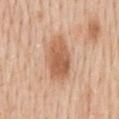Q: Was this lesion biopsied?
A: no biopsy performed (imaged during a skin exam)
Q: Patient demographics?
A: male, aged 58 to 62
Q: What is the imaging modality?
A: 15 mm crop, total-body photography
Q: What is the lesion's diameter?
A: ~5 mm (longest diameter)
Q: Automated lesion metrics?
A: a border-irregularity index near 2/10, a within-lesion color-variation index near 3.5/10, and radial color variation of about 1; a nevus-likeness score of about 95/100 and a detector confidence of about 100 out of 100 that the crop contains a lesion
Q: What lighting was used for the tile?
A: white-light
Q: Where on the body is the lesion?
A: the mid back